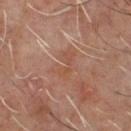notes = catalogued during a skin exam; not biopsied
tile lighting = cross-polarized illumination
location = the front of the torso
automated metrics = a mean CIELAB color near L≈46 a*≈20 b*≈28
acquisition = ~15 mm tile from a whole-body skin photo
lesion diameter = about 3.5 mm
subject = male, approximately 60 years of age